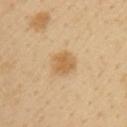image: 15 mm crop, total-body photography
lesion diameter: about 3.5 mm
lighting: cross-polarized
TBP lesion metrics: a footprint of about 6.5 mm² and a shape-asymmetry score of about 0.2 (0 = symmetric)
subject: male, in their 40s
body site: the left upper arm